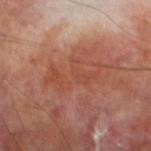{
  "biopsy_status": "not biopsied; imaged during a skin examination",
  "site": "leg",
  "automated_metrics": {
    "lesion_detection_confidence_0_100": 95
  },
  "image": {
    "source": "total-body photography crop",
    "field_of_view_mm": 15
  },
  "patient": {
    "sex": "male",
    "age_approx": 70
  }
}